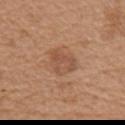The lesion was tiled from a total-body skin photograph and was not biopsied. A female patient, aged 53 to 57. Measured at roughly 3.5 mm in maximum diameter. A roughly 15 mm field-of-view crop from a total-body skin photograph. Located on the right upper arm.